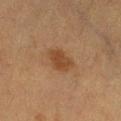workup = catalogued during a skin exam; not biopsied
automated metrics = a footprint of about 6 mm² and two-axis asymmetry of about 0.25; a mean CIELAB color near L≈36 a*≈18 b*≈29; a lesion-detection confidence of about 100/100
lesion diameter = ≈3 mm
image source = ~15 mm tile from a whole-body skin photo
illumination = cross-polarized illumination
subject = female, in their mid-50s
body site = the leg